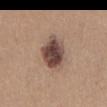{"image": {"source": "total-body photography crop", "field_of_view_mm": 15}, "patient": {"sex": "male", "age_approx": 25}, "site": "front of the torso"}